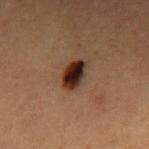Impression:
Part of a total-body skin-imaging series; this lesion was reviewed on a skin check and was not flagged for biopsy.
Clinical summary:
On the mid back. The subject is a male aged 48 to 52. A roughly 15 mm field-of-view crop from a total-body skin photograph. Longest diameter approximately 3.5 mm.